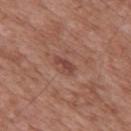This lesion was catalogued during total-body skin photography and was not selected for biopsy. A male patient about 50 years old. The tile uses white-light illumination. A close-up tile cropped from a whole-body skin photograph, about 15 mm across. From the mid back. Approximately 2.5 mm at its widest.